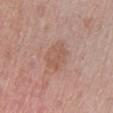A 15 mm close-up extracted from a 3D total-body photography capture. This is a white-light tile. Located on the left lower leg. Longest diameter approximately 4 mm. The lesion-visualizer software estimated a footprint of about 9 mm², a shape eccentricity near 0.6, and a symmetry-axis asymmetry near 0.25. The analysis additionally found a lesion color around L≈56 a*≈20 b*≈27 in CIELAB. The analysis additionally found a within-lesion color-variation index near 2.5/10. A female patient about 65 years old.